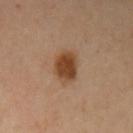<tbp_lesion>
  <biopsy_status>not biopsied; imaged during a skin examination</biopsy_status>
  <lighting>cross-polarized</lighting>
  <patient>
    <sex>female</sex>
    <age_approx>40</age_approx>
  </patient>
  <lesion_size>
    <long_diameter_mm_approx>3.5</long_diameter_mm_approx>
  </lesion_size>
  <site>left upper arm</site>
  <image>
    <source>total-body photography crop</source>
    <field_of_view_mm>15</field_of_view_mm>
  </image>
</tbp_lesion>